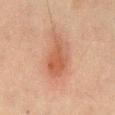lesion size = about 6 mm
image-analysis metrics = a footprint of about 13 mm², an eccentricity of roughly 0.85, and two-axis asymmetry of about 0.3; an average lesion color of about L≈46 a*≈21 b*≈28 (CIELAB), about 8 CIELAB-L* units darker than the surrounding skin, and a normalized lesion–skin contrast near 6.5
lighting = cross-polarized illumination
patient = male, about 65 years old
body site = the front of the torso
image = ~15 mm crop, total-body skin-cancer survey